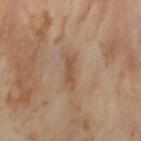The lesion was tiled from a total-body skin photograph and was not biopsied.
Automated tile analysis of the lesion measured a lesion area of about 5 mm², a shape eccentricity near 0.95, and a symmetry-axis asymmetry near 0.4. And it measured a border-irregularity index near 5/10, a within-lesion color-variation index near 2/10, and radial color variation of about 0.5.
Captured under cross-polarized illumination.
About 4 mm across.
A female patient, aged 53 to 57.
A lesion tile, about 15 mm wide, cut from a 3D total-body photograph.
The lesion is on the leg.